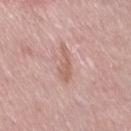{"biopsy_status": "not biopsied; imaged during a skin examination", "image": {"source": "total-body photography crop", "field_of_view_mm": 15}, "site": "leg", "patient": {"sex": "female", "age_approx": 40}}